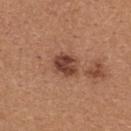Q: What are the patient's age and sex?
A: female, roughly 40 years of age
Q: How was this image acquired?
A: 15 mm crop, total-body photography
Q: Lesion size?
A: ~3.5 mm (longest diameter)
Q: Lesion location?
A: the upper back